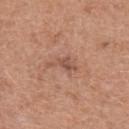Located on the upper back. About 4 mm across. A female patient, roughly 60 years of age. The total-body-photography lesion software estimated a mean CIELAB color near L≈52 a*≈21 b*≈29, a lesion–skin lightness drop of about 8, and a lesion-to-skin contrast of about 6 (normalized; higher = more distinct). The tile uses white-light illumination. This image is a 15 mm lesion crop taken from a total-body photograph.